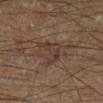No biopsy was performed on this lesion — it was imaged during a full skin examination and was not determined to be concerning.
Imaged with cross-polarized lighting.
On the right lower leg.
The patient is a male aged approximately 65.
A close-up tile cropped from a whole-body skin photograph, about 15 mm across.
Measured at roughly 4 mm in maximum diameter.